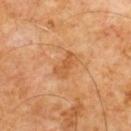workup: no biopsy performed (imaged during a skin exam)
TBP lesion metrics: border irregularity of about 4 on a 0–10 scale, internal color variation of about 2 on a 0–10 scale, and peripheral color asymmetry of about 0.5
site: the front of the torso
subject: male, roughly 60 years of age
lighting: cross-polarized
diameter: about 3 mm
acquisition: total-body-photography crop, ~15 mm field of view